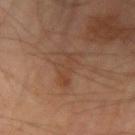Case summary:
* anatomic site: the left forearm
* subject: male, aged 68 to 72
* illumination: cross-polarized
* image: ~15 mm crop, total-body skin-cancer survey
* size: ~4.5 mm (longest diameter)
* image-analysis metrics: a lesion area of about 7 mm², an eccentricity of roughly 0.9, and a symmetry-axis asymmetry near 0.5